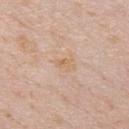follow-up — catalogued during a skin exam; not biopsied | patient — male, aged 48 to 52 | acquisition — 15 mm crop, total-body photography | diameter — about 2.5 mm | automated metrics — a border-irregularity index near 2.5/10, internal color variation of about 2.5 on a 0–10 scale, and peripheral color asymmetry of about 1 | location — the chest.